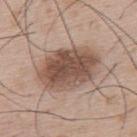The lesion was tiled from a total-body skin photograph and was not biopsied. A male patient, aged 53 to 57. This is a white-light tile. A 15 mm close-up tile from a total-body photography series done for melanoma screening. From the upper back.